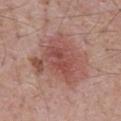follow-up=total-body-photography surveillance lesion; no biopsy | size=about 7.5 mm | imaging modality=~15 mm crop, total-body skin-cancer survey | site=the chest | subject=male, about 70 years old.